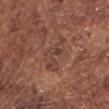Impression: The lesion was tiled from a total-body skin photograph and was not biopsied. Acquisition and patient details: The recorded lesion diameter is about 5.5 mm. This is a white-light tile. A 15 mm crop from a total-body photograph taken for skin-cancer surveillance. A male patient in their mid- to late 70s. Automated image analysis of the tile measured a footprint of about 8.5 mm², an outline eccentricity of about 0.9 (0 = round, 1 = elongated), and a symmetry-axis asymmetry near 0.55. It also reported a lesion–skin lightness drop of about 6 and a normalized border contrast of about 5.5. The analysis additionally found a color-variation rating of about 5/10 and radial color variation of about 2. The software also gave a classifier nevus-likeness of about 5/100. From the chest.